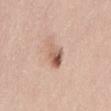| key | value |
|---|---|
| follow-up | catalogued during a skin exam; not biopsied |
| patient | female, approximately 50 years of age |
| image | total-body-photography crop, ~15 mm field of view |
| body site | the mid back |